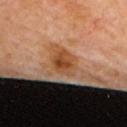Q: Was a biopsy performed?
A: no biopsy performed (imaged during a skin exam)
Q: Patient demographics?
A: female, aged 63–67
Q: What lighting was used for the tile?
A: cross-polarized
Q: What is the lesion's diameter?
A: ~3.5 mm (longest diameter)
Q: What kind of image is this?
A: ~15 mm tile from a whole-body skin photo
Q: Where on the body is the lesion?
A: the upper back